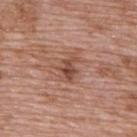Notes:
• follow-up — no biopsy performed (imaged during a skin exam)
• lighting — white-light illumination
• size — about 3 mm
• automated lesion analysis — a footprint of about 4.5 mm², a shape eccentricity near 0.7, and a symmetry-axis asymmetry near 0.35; an average lesion color of about L≈47 a*≈23 b*≈28 (CIELAB)
• subject — male, aged 68–72
• image source — total-body-photography crop, ~15 mm field of view
• location — the upper back